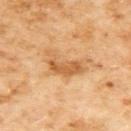Imaged during a routine full-body skin examination; the lesion was not biopsied and no histopathology is available. The tile uses cross-polarized illumination. A female patient aged around 60. On the upper back. The lesion's longest dimension is about 5.5 mm. Cropped from a whole-body photographic skin survey; the tile spans about 15 mm.